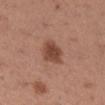follow-up — total-body-photography surveillance lesion; no biopsy
automated lesion analysis — a footprint of about 7 mm² and an outline eccentricity of about 0.55 (0 = round, 1 = elongated); border irregularity of about 2.5 on a 0–10 scale, internal color variation of about 2.5 on a 0–10 scale, and radial color variation of about 0.5; an automated nevus-likeness rating near 90 out of 100
lesion size — ≈3 mm
site — the right lower leg
subject — female, in their 30s
imaging modality — ~15 mm tile from a whole-body skin photo
illumination — white-light illumination